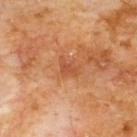biopsy status = catalogued during a skin exam; not biopsied | image = 15 mm crop, total-body photography | TBP lesion metrics = a border-irregularity rating of about 4/10, a within-lesion color-variation index near 1/10, and radial color variation of about 0; an automated nevus-likeness rating near 0 out of 100 and a detector confidence of about 100 out of 100 that the crop contains a lesion | tile lighting = cross-polarized | lesion diameter = ~3 mm (longest diameter) | patient = male, roughly 60 years of age | body site = the front of the torso.